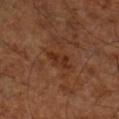Notes:
• notes — catalogued during a skin exam; not biopsied
• lesion diameter — about 3 mm
• automated lesion analysis — an automated nevus-likeness rating near 0 out of 100 and lesion-presence confidence of about 100/100
• location — the right lower leg
• tile lighting — cross-polarized
• subject — aged around 65
• acquisition — 15 mm crop, total-body photography